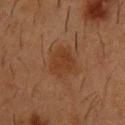Impression:
The lesion was tiled from a total-body skin photograph and was not biopsied.